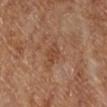notes: no biopsy performed (imaged during a skin exam)
subject: female
lesion diameter: about 2.5 mm
automated lesion analysis: an area of roughly 4 mm² and two-axis asymmetry of about 0.3; an average lesion color of about L≈46 a*≈22 b*≈32 (CIELAB) and a lesion–skin lightness drop of about 7; border irregularity of about 3.5 on a 0–10 scale, a color-variation rating of about 1/10, and a peripheral color-asymmetry measure near 0.5; a nevus-likeness score of about 0/100 and a detector confidence of about 100 out of 100 that the crop contains a lesion
lighting: cross-polarized illumination
anatomic site: the leg
image source: ~15 mm crop, total-body skin-cancer survey